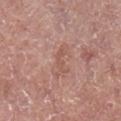Q: Is there a histopathology result?
A: total-body-photography surveillance lesion; no biopsy
Q: Where on the body is the lesion?
A: the right lower leg
Q: How was this image acquired?
A: 15 mm crop, total-body photography
Q: How was the tile lit?
A: white-light
Q: Who is the patient?
A: male, aged around 55
Q: Automated lesion metrics?
A: two-axis asymmetry of about 0.55; border irregularity of about 6 on a 0–10 scale, internal color variation of about 0 on a 0–10 scale, and radial color variation of about 0; a classifier nevus-likeness of about 0/100 and a detector confidence of about 100 out of 100 that the crop contains a lesion